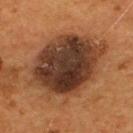Notes:
• notes — imaged on a skin check; not biopsied
• image — ~15 mm crop, total-body skin-cancer survey
• size — ~10 mm (longest diameter)
• subject — male, in their mid-50s
• body site — the back